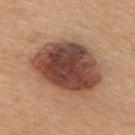Captured during whole-body skin photography for melanoma surveillance; the lesion was not biopsied.
The lesion's longest dimension is about 8 mm.
Located on the upper back.
A female patient, in their 30s.
A region of skin cropped from a whole-body photographic capture, roughly 15 mm wide.